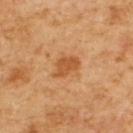Assessment: The lesion was photographed on a routine skin check and not biopsied; there is no pathology result. Clinical summary: From the upper back. Approximately 4 mm at its widest. This is a cross-polarized tile. The patient is a male aged 58 to 62. A close-up tile cropped from a whole-body skin photograph, about 15 mm across.